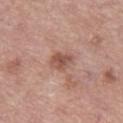Acquisition and patient details: Cropped from a total-body skin-imaging series; the visible field is about 15 mm. The lesion is located on the left thigh. A male subject, in their mid-50s.